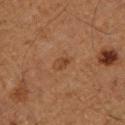Captured during whole-body skin photography for melanoma surveillance; the lesion was not biopsied.
The subject is a male in their mid- to late 70s.
This is a cross-polarized tile.
The lesion's longest dimension is about 3 mm.
The lesion is on the left lower leg.
A roughly 15 mm field-of-view crop from a total-body skin photograph.